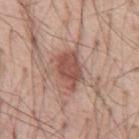Assessment:
Recorded during total-body skin imaging; not selected for excision or biopsy.
Image and clinical context:
Cropped from a total-body skin-imaging series; the visible field is about 15 mm. Located on the front of the torso. Imaged with white-light lighting. A male patient, aged 53–57. Longest diameter approximately 4.5 mm.